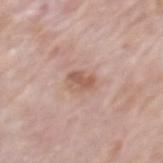Part of a total-body skin-imaging series; this lesion was reviewed on a skin check and was not flagged for biopsy. From the mid back. A 15 mm crop from a total-body photograph taken for skin-cancer surveillance. A male patient roughly 80 years of age. The recorded lesion diameter is about 3.5 mm.